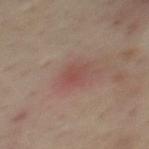Assessment: Imaged during a routine full-body skin examination; the lesion was not biopsied and no histopathology is available. Context: From the mid back. A roughly 15 mm field-of-view crop from a total-body skin photograph. Automated image analysis of the tile measured an area of roughly 4 mm², an outline eccentricity of about 0.65 (0 = round, 1 = elongated), and a shape-asymmetry score of about 0.25 (0 = symmetric). The software also gave a lesion color around L≈45 a*≈22 b*≈22 in CIELAB, a lesion–skin lightness drop of about 6, and a normalized lesion–skin contrast near 5. The software also gave an automated nevus-likeness rating near 0 out of 100 and lesion-presence confidence of about 100/100. Longest diameter approximately 2.5 mm. The subject is a male aged around 45.